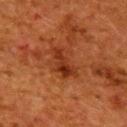follow-up: no biopsy performed (imaged during a skin exam)
acquisition: 15 mm crop, total-body photography
patient: female, approximately 50 years of age
image-analysis metrics: a footprint of about 6.5 mm², a shape eccentricity near 0.9, and two-axis asymmetry of about 0.4
lighting: cross-polarized illumination
size: about 4.5 mm
site: the upper back